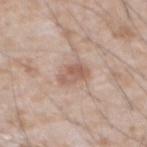Case summary:
* biopsy status: imaged on a skin check; not biopsied
* image: ~15 mm crop, total-body skin-cancer survey
* patient: male, aged around 65
* site: the abdomen
* lesion diameter: ~3 mm (longest diameter)
* tile lighting: white-light illumination